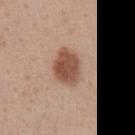Q: Was a biopsy performed?
A: catalogued during a skin exam; not biopsied
Q: Illumination type?
A: white-light
Q: Lesion location?
A: the front of the torso
Q: Automated lesion metrics?
A: lesion-presence confidence of about 100/100
Q: What is the imaging modality?
A: total-body-photography crop, ~15 mm field of view
Q: How large is the lesion?
A: about 4.5 mm
Q: What are the patient's age and sex?
A: female, aged around 45